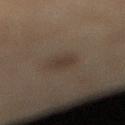Q: Was a biopsy performed?
A: catalogued during a skin exam; not biopsied
Q: Lesion size?
A: ≈2.5 mm
Q: Who is the patient?
A: male, in their mid- to late 80s or older
Q: Lesion location?
A: the right lower leg
Q: Automated lesion metrics?
A: a footprint of about 4.5 mm², an eccentricity of roughly 0.65, and a symmetry-axis asymmetry near 0.25; an average lesion color of about L≈29 a*≈10 b*≈19 (CIELAB), about 6 CIELAB-L* units darker than the surrounding skin, and a normalized lesion–skin contrast near 6; a border-irregularity rating of about 2.5/10, a color-variation rating of about 1/10, and peripheral color asymmetry of about 0.5
Q: How was this image acquired?
A: ~15 mm tile from a whole-body skin photo